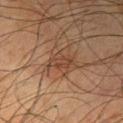{
  "biopsy_status": "not biopsied; imaged during a skin examination",
  "patient": {
    "sex": "male",
    "age_approx": 75
  },
  "lesion_size": {
    "long_diameter_mm_approx": 3.0
  },
  "image": {
    "source": "total-body photography crop",
    "field_of_view_mm": 15
  },
  "site": "arm",
  "lighting": "cross-polarized"
}